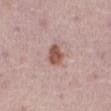Impression: Captured during whole-body skin photography for melanoma surveillance; the lesion was not biopsied. Context: A 15 mm close-up tile from a total-body photography series done for melanoma screening. The tile uses white-light illumination. The lesion is located on the abdomen. A male subject aged 73 to 77.